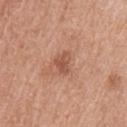Part of a total-body skin-imaging series; this lesion was reviewed on a skin check and was not flagged for biopsy.
The tile uses white-light illumination.
The lesion's longest dimension is about 3 mm.
An algorithmic analysis of the crop reported an area of roughly 4 mm² and an outline eccentricity of about 0.7 (0 = round, 1 = elongated). The analysis additionally found a lesion–skin lightness drop of about 9 and a normalized lesion–skin contrast near 6.5. And it measured internal color variation of about 2 on a 0–10 scale and peripheral color asymmetry of about 0.5. It also reported a classifier nevus-likeness of about 0/100 and a detector confidence of about 100 out of 100 that the crop contains a lesion.
A 15 mm close-up tile from a total-body photography series done for melanoma screening.
The subject is a male aged approximately 60.
Located on the upper back.